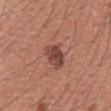Clinical impression:
Part of a total-body skin-imaging series; this lesion was reviewed on a skin check and was not flagged for biopsy.
Background:
On the mid back. Automated image analysis of the tile measured a mean CIELAB color near L≈45 a*≈24 b*≈25 and a normalized border contrast of about 8.5. And it measured a nevus-likeness score of about 75/100. A male subject, aged around 45. Imaged with white-light lighting. The lesion's longest dimension is about 3.5 mm. A 15 mm crop from a total-body photograph taken for skin-cancer surveillance.